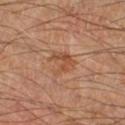Q: Is there a histopathology result?
A: catalogued during a skin exam; not biopsied
Q: Where on the body is the lesion?
A: the right lower leg
Q: Who is the patient?
A: male, about 70 years old
Q: What is the imaging modality?
A: total-body-photography crop, ~15 mm field of view
Q: How was the tile lit?
A: cross-polarized illumination
Q: How large is the lesion?
A: ≈3.5 mm